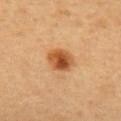Imaged during a routine full-body skin examination; the lesion was not biopsied and no histopathology is available.